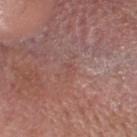This lesion was catalogued during total-body skin photography and was not selected for biopsy.
Imaged with white-light lighting.
The lesion is on the head or neck.
Longest diameter approximately 1.5 mm.
A roughly 15 mm field-of-view crop from a total-body skin photograph.
The subject is a female aged around 65.